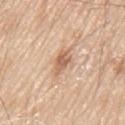The subject is a male aged 78–82. A lesion tile, about 15 mm wide, cut from a 3D total-body photograph. Located on the mid back.